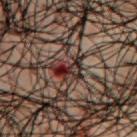Impression:
Recorded during total-body skin imaging; not selected for excision or biopsy.
Acquisition and patient details:
A region of skin cropped from a whole-body photographic capture, roughly 15 mm wide. The recorded lesion diameter is about 3.5 mm. The patient is a male aged 48–52. Captured under cross-polarized illumination. Automated image analysis of the tile measured a mean CIELAB color near L≈21 a*≈16 b*≈16, roughly 10 lightness units darker than nearby skin, and a normalized border contrast of about 11.5. It also reported a border-irregularity rating of about 5.5/10, a within-lesion color-variation index near 10/10, and a peripheral color-asymmetry measure near 4. The software also gave a nevus-likeness score of about 0/100 and lesion-presence confidence of about 100/100. On the chest.